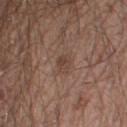Assessment: This lesion was catalogued during total-body skin photography and was not selected for biopsy. Image and clinical context: A region of skin cropped from a whole-body photographic capture, roughly 15 mm wide. A male patient in their 20s. Located on the right thigh. This is a white-light tile. Automated image analysis of the tile measured a footprint of about 3.5 mm², a shape eccentricity near 0.8, and a shape-asymmetry score of about 0.25 (0 = symmetric). The software also gave about 7 CIELAB-L* units darker than the surrounding skin and a lesion-to-skin contrast of about 6 (normalized; higher = more distinct). And it measured a within-lesion color-variation index near 2/10 and radial color variation of about 1.